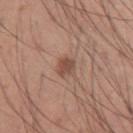Captured during whole-body skin photography for melanoma surveillance; the lesion was not biopsied. Automated image analysis of the tile measured a footprint of about 4 mm², an outline eccentricity of about 0.7 (0 = round, 1 = elongated), and a shape-asymmetry score of about 0.2 (0 = symmetric). It also reported a lesion color around L≈49 a*≈21 b*≈26 in CIELAB, a lesion–skin lightness drop of about 10, and a normalized border contrast of about 7.5. The analysis additionally found a border-irregularity index near 2/10, a within-lesion color-variation index near 2/10, and peripheral color asymmetry of about 0.5. Cropped from a whole-body photographic skin survey; the tile spans about 15 mm. Captured under white-light illumination. A male patient, in their mid-30s. From the left upper arm.